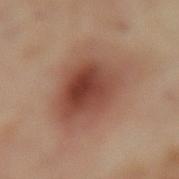| key | value |
|---|---|
| patient | female, in their mid- to late 50s |
| body site | the lower back |
| image | ~15 mm tile from a whole-body skin photo |
| lighting | cross-polarized |
| lesion diameter | about 7.5 mm |